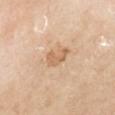This lesion was catalogued during total-body skin photography and was not selected for biopsy. A roughly 15 mm field-of-view crop from a total-body skin photograph. The tile uses cross-polarized illumination. The recorded lesion diameter is about 3 mm. The patient is a male aged around 55. On the leg.